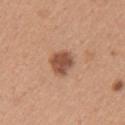Impression: Recorded during total-body skin imaging; not selected for excision or biopsy. Image and clinical context: A 15 mm close-up tile from a total-body photography series done for melanoma screening. The subject is a female roughly 30 years of age. From the left upper arm.